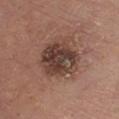<lesion>
  <automated_metrics>
    <area_mm2_approx>17.0</area_mm2_approx>
    <eccentricity>0.5</eccentricity>
  </automated_metrics>
  <image>
    <source>total-body photography crop</source>
    <field_of_view_mm>15</field_of_view_mm>
  </image>
  <patient>
    <sex>male</sex>
    <age_approx>80</age_approx>
  </patient>
  <lesion_size>
    <long_diameter_mm_approx>5.0</long_diameter_mm_approx>
  </lesion_size>
  <site>chest</site>
</lesion>